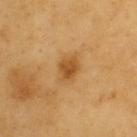* notes · no biopsy performed (imaged during a skin exam)
* subject · male, aged 58 to 62
* image · ~15 mm crop, total-body skin-cancer survey
* body site · the upper back
* illumination · cross-polarized illumination
* lesion size · ≈3 mm